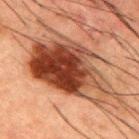Imaged during a routine full-body skin examination; the lesion was not biopsied and no histopathology is available.
A 15 mm close-up tile from a total-body photography series done for melanoma screening.
On the upper back.
The tile uses cross-polarized illumination.
A male patient, in their 50s.
About 11.5 mm across.